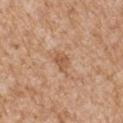<tbp_lesion>
<biopsy_status>not biopsied; imaged during a skin examination</biopsy_status>
<patient>
  <sex>male</sex>
  <age_approx>65</age_approx>
</patient>
<image>
  <source>total-body photography crop</source>
  <field_of_view_mm>15</field_of_view_mm>
</image>
<lighting>white-light</lighting>
<site>left upper arm</site>
<lesion_size>
  <long_diameter_mm_approx>3.0</long_diameter_mm_approx>
</lesion_size>
</tbp_lesion>